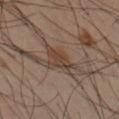follow-up = no biopsy performed (imaged during a skin exam); image source = 15 mm crop, total-body photography; anatomic site = the left forearm; size = ≈4 mm; patient = male, aged around 40.